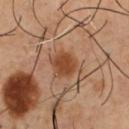automated lesion analysis: an average lesion color of about L≈45 a*≈22 b*≈33 (CIELAB) and a lesion-to-skin contrast of about 8 (normalized; higher = more distinct); a border-irregularity index near 2/10, internal color variation of about 2.5 on a 0–10 scale, and peripheral color asymmetry of about 0.5; a classifier nevus-likeness of about 75/100 and a detector confidence of about 100 out of 100 that the crop contains a lesion
subject: male, in their mid- to late 50s
illumination: cross-polarized illumination
image source: ~15 mm tile from a whole-body skin photo
lesion size: about 4 mm
location: the chest